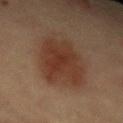notes: imaged on a skin check; not biopsied | subject: female, about 60 years old | automated metrics: a lesion area of about 25 mm²; border irregularity of about 3 on a 0–10 scale, a within-lesion color-variation index near 4/10, and a peripheral color-asymmetry measure near 1.5; a nevus-likeness score of about 95/100 and a lesion-detection confidence of about 100/100 | image source: 15 mm crop, total-body photography | diameter: ≈7 mm | anatomic site: the abdomen.